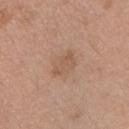{"biopsy_status": "not biopsied; imaged during a skin examination", "automated_metrics": {"eccentricity": 0.8, "shape_asymmetry": 0.2, "border_irregularity_0_10": 2.5, "color_variation_0_10": 2.0, "peripheral_color_asymmetry": 1.0}, "patient": {"sex": "male", "age_approx": 30}, "site": "chest", "lighting": "white-light", "image": {"source": "total-body photography crop", "field_of_view_mm": 15}}